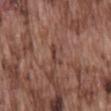<record>
  <biopsy_status>not biopsied; imaged during a skin examination</biopsy_status>
  <patient>
    <sex>male</sex>
    <age_approx>75</age_approx>
  </patient>
  <lighting>white-light</lighting>
  <site>back</site>
  <lesion_size>
    <long_diameter_mm_approx>3.0</long_diameter_mm_approx>
  </lesion_size>
  <image>
    <source>total-body photography crop</source>
    <field_of_view_mm>15</field_of_view_mm>
  </image>
</record>